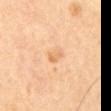notes: total-body-photography surveillance lesion; no biopsy